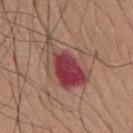The lesion was tiled from a total-body skin photograph and was not biopsied. Approximately 7 mm at its widest. The lesion is on the chest. Imaged with white-light lighting. The patient is a male about 65 years old. A close-up tile cropped from a whole-body skin photograph, about 15 mm across. The total-body-photography lesion software estimated an eccentricity of roughly 0.85 and two-axis asymmetry of about 0.5.